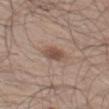The lesion was tiled from a total-body skin photograph and was not biopsied. A 15 mm close-up tile from a total-body photography series done for melanoma screening. The lesion is located on the left thigh. Measured at roughly 3 mm in maximum diameter. Automated image analysis of the tile measured border irregularity of about 2.5 on a 0–10 scale and a peripheral color-asymmetry measure near 1. A male subject, aged around 60.